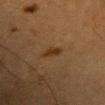Part of a total-body skin-imaging series; this lesion was reviewed on a skin check and was not flagged for biopsy. Imaged with cross-polarized lighting. Located on the head or neck. A 15 mm close-up tile from a total-body photography series done for melanoma screening. A female patient, aged around 40.